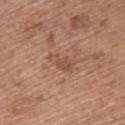Assessment:
The lesion was photographed on a routine skin check and not biopsied; there is no pathology result.
Context:
The lesion is located on the upper back. A 15 mm close-up extracted from a 3D total-body photography capture. Longest diameter approximately 3.5 mm. This is a white-light tile. An algorithmic analysis of the crop reported a nevus-likeness score of about 0/100 and a detector confidence of about 100 out of 100 that the crop contains a lesion. A female patient roughly 60 years of age.